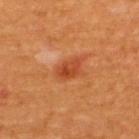<tbp_lesion>
<biopsy_status>not biopsied; imaged during a skin examination</biopsy_status>
<patient>
  <sex>male</sex>
</patient>
<lighting>cross-polarized</lighting>
<site>upper back</site>
<image>
  <source>total-body photography crop</source>
  <field_of_view_mm>15</field_of_view_mm>
</image>
<lesion_size>
  <long_diameter_mm_approx>3.0</long_diameter_mm_approx>
</lesion_size>
</tbp_lesion>